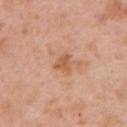Notes:
* biopsy status: total-body-photography surveillance lesion; no biopsy
* image: 15 mm crop, total-body photography
* site: the left upper arm
* subject: female, aged around 40
* lighting: white-light illumination